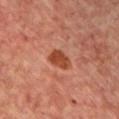– follow-up: total-body-photography surveillance lesion; no biopsy
– image-analysis metrics: border irregularity of about 2 on a 0–10 scale and a color-variation rating of about 2.5/10; a nevus-likeness score of about 85/100 and a lesion-detection confidence of about 100/100
– image: total-body-photography crop, ~15 mm field of view
– illumination: cross-polarized illumination
– anatomic site: the chest
– size: about 3 mm
– subject: female, about 45 years old